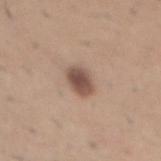Recorded during total-body skin imaging; not selected for excision or biopsy. About 3 mm across. The tile uses white-light illumination. Automated tile analysis of the lesion measured an automated nevus-likeness rating near 95 out of 100 and a detector confidence of about 100 out of 100 that the crop contains a lesion. Cropped from a total-body skin-imaging series; the visible field is about 15 mm. The subject is a male aged 58 to 62. On the abdomen.